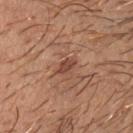No biopsy was performed on this lesion — it was imaged during a full skin examination and was not determined to be concerning.
A male patient aged 38–42.
On the head or neck.
Cropped from a total-body skin-imaging series; the visible field is about 15 mm.